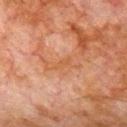No biopsy was performed on this lesion — it was imaged during a full skin examination and was not determined to be concerning. The recorded lesion diameter is about 2.5 mm. A roughly 15 mm field-of-view crop from a total-body skin photograph. The patient is a male aged 78 to 82. On the chest. Imaged with cross-polarized lighting.